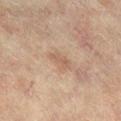This lesion was catalogued during total-body skin photography and was not selected for biopsy.
From the right lower leg.
A female subject about 80 years old.
Cropped from a whole-body photographic skin survey; the tile spans about 15 mm.